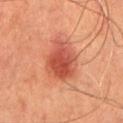Image and clinical context:
Approximately 5 mm at its widest. The lesion is on the chest. A male patient, aged approximately 65. Cropped from a total-body skin-imaging series; the visible field is about 15 mm. This is a cross-polarized tile.